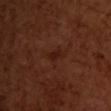Assessment: This lesion was catalogued during total-body skin photography and was not selected for biopsy. Context: The total-body-photography lesion software estimated an area of roughly 3 mm², an eccentricity of roughly 0.9, and a symmetry-axis asymmetry near 0.4. And it measured a lesion–skin lightness drop of about 5 and a normalized border contrast of about 6.5. Located on the upper back. A female subject in their mid-50s. Approximately 3 mm at its widest. A 15 mm close-up tile from a total-body photography series done for melanoma screening.